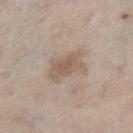Impression: This lesion was catalogued during total-body skin photography and was not selected for biopsy. Image and clinical context: The recorded lesion diameter is about 4.5 mm. Cropped from a total-body skin-imaging series; the visible field is about 15 mm. The lesion is located on the right lower leg. A male subject, in their 60s. This is a white-light tile.